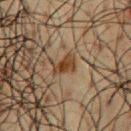Q: Was this lesion biopsied?
A: catalogued during a skin exam; not biopsied
Q: Patient demographics?
A: male, aged around 60
Q: What is the lesion's diameter?
A: about 3 mm
Q: Where on the body is the lesion?
A: the chest
Q: What lighting was used for the tile?
A: cross-polarized illumination
Q: How was this image acquired?
A: 15 mm crop, total-body photography